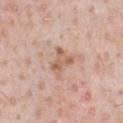Impression: Recorded during total-body skin imaging; not selected for excision or biopsy. Context: The patient is a male aged around 50. Captured under white-light illumination. On the chest. Cropped from a total-body skin-imaging series; the visible field is about 15 mm. Longest diameter approximately 3 mm.